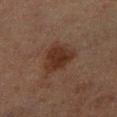| feature | finding |
|---|---|
| follow-up | no biopsy performed (imaged during a skin exam) |
| anatomic site | the left lower leg |
| imaging modality | ~15 mm crop, total-body skin-cancer survey |
| patient | male, approximately 65 years of age |
| lesion diameter | ≈4 mm |